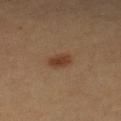Clinical impression:
This lesion was catalogued during total-body skin photography and was not selected for biopsy.
Background:
The lesion is on the left lower leg. Measured at roughly 3 mm in maximum diameter. A roughly 15 mm field-of-view crop from a total-body skin photograph. This is a cross-polarized tile. A female subject aged around 60.